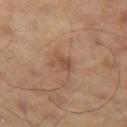{"site": "left thigh", "lighting": "cross-polarized", "automated_metrics": {"eccentricity": 0.85, "shape_asymmetry": 0.4, "cielab_L": 50, "cielab_a": 21, "cielab_b": 30, "vs_skin_darker_L": 8.0, "vs_skin_contrast_norm": 6.0, "nevus_likeness_0_100": 0, "lesion_detection_confidence_0_100": 100}, "lesion_size": {"long_diameter_mm_approx": 2.5}, "image": {"source": "total-body photography crop", "field_of_view_mm": 15}}